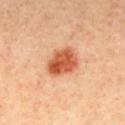The lesion was tiled from a total-body skin photograph and was not biopsied. A male subject aged 38 to 42. On the mid back. The lesion-visualizer software estimated a footprint of about 11 mm² and two-axis asymmetry of about 0.2. And it measured a lesion color around L≈59 a*≈32 b*≈41 in CIELAB and about 16 CIELAB-L* units darker than the surrounding skin. And it measured border irregularity of about 2 on a 0–10 scale and internal color variation of about 5.5 on a 0–10 scale. Captured under cross-polarized illumination. About 4 mm across. A 15 mm close-up extracted from a 3D total-body photography capture.